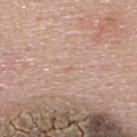Part of a total-body skin-imaging series; this lesion was reviewed on a skin check and was not flagged for biopsy. The recorded lesion diameter is about 1.5 mm. The tile uses white-light illumination. A male subject, in their mid-50s. The lesion is on the upper back. Cropped from a total-body skin-imaging series; the visible field is about 15 mm. Automated image analysis of the tile measured a border-irregularity rating of about 4.5/10, a color-variation rating of about 0/10, and a peripheral color-asymmetry measure near 0. It also reported a nevus-likeness score of about 0/100 and a detector confidence of about 90 out of 100 that the crop contains a lesion.